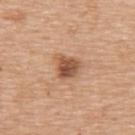Acquisition and patient details:
A close-up tile cropped from a whole-body skin photograph, about 15 mm across. The subject is a female about 55 years old. The lesion is located on the upper back.